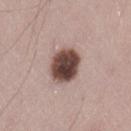Q: Is there a histopathology result?
A: no biopsy performed (imaged during a skin exam)
Q: What lighting was used for the tile?
A: white-light
Q: Where on the body is the lesion?
A: the left thigh
Q: What is the imaging modality?
A: ~15 mm crop, total-body skin-cancer survey
Q: What did automated image analysis measure?
A: a mean CIELAB color near L≈45 a*≈18 b*≈21 and a lesion–skin lightness drop of about 21; an automated nevus-likeness rating near 40 out of 100 and lesion-presence confidence of about 100/100
Q: Who is the patient?
A: male, roughly 45 years of age